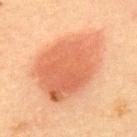| field | value |
|---|---|
| follow-up | imaged on a skin check; not biopsied |
| acquisition | ~15 mm crop, total-body skin-cancer survey |
| patient | male, aged around 60 |
| image-analysis metrics | a lesion area of about 49 mm² and a shape-asymmetry score of about 0.2 (0 = symmetric); border irregularity of about 2.5 on a 0–10 scale, internal color variation of about 5 on a 0–10 scale, and peripheral color asymmetry of about 1.5; a classifier nevus-likeness of about 100/100 and lesion-presence confidence of about 100/100 |
| size | about 9.5 mm |
| lighting | cross-polarized |
| location | the upper back |